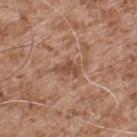Assessment:
This lesion was catalogued during total-body skin photography and was not selected for biopsy.
Clinical summary:
This is a white-light tile. A lesion tile, about 15 mm wide, cut from a 3D total-body photograph. The patient is a male approximately 55 years of age. Located on the upper back.